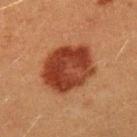<lesion>
<biopsy_status>not biopsied; imaged during a skin examination</biopsy_status>
<lesion_size>
  <long_diameter_mm_approx>6.0</long_diameter_mm_approx>
</lesion_size>
<image>
  <source>total-body photography crop</source>
  <field_of_view_mm>15</field_of_view_mm>
</image>
<patient>
  <sex>male</sex>
  <age_approx>40</age_approx>
</patient>
<lighting>cross-polarized</lighting>
<site>left upper arm</site>
</lesion>